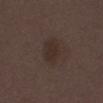Recorded during total-body skin imaging; not selected for excision or biopsy.
Automated image analysis of the tile measured an eccentricity of roughly 0.8 and a shape-asymmetry score of about 0.15 (0 = symmetric). The analysis additionally found an automated nevus-likeness rating near 15 out of 100 and lesion-presence confidence of about 100/100.
The patient is a male in their 50s.
The lesion's longest dimension is about 3.5 mm.
This is a white-light tile.
A roughly 15 mm field-of-view crop from a total-body skin photograph.